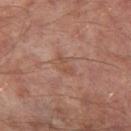biopsy status: no biopsy performed (imaged during a skin exam).